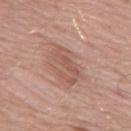Context: Approximately 5 mm at its widest. The lesion is located on the leg. A region of skin cropped from a whole-body photographic capture, roughly 15 mm wide. This is a white-light tile. A female patient, roughly 70 years of age.